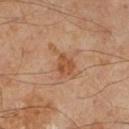Assessment: The lesion was tiled from a total-body skin photograph and was not biopsied. Acquisition and patient details: Imaged with cross-polarized lighting. Located on the right lower leg. The total-body-photography lesion software estimated a footprint of about 8 mm² and a shape eccentricity near 0.8. The software also gave an average lesion color of about L≈46 a*≈20 b*≈31 (CIELAB) and roughly 7 lightness units darker than nearby skin. It also reported an automated nevus-likeness rating near 0 out of 100 and a detector confidence of about 100 out of 100 that the crop contains a lesion. The subject is a male aged around 60. A 15 mm close-up extracted from a 3D total-body photography capture. The lesion's longest dimension is about 4.5 mm.